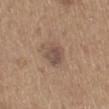Case summary:
• site: the mid back
• tile lighting: white-light
• patient: male, roughly 65 years of age
• lesion diameter: ~2.5 mm (longest diameter)
• image source: 15 mm crop, total-body photography